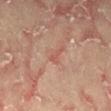workup = no biopsy performed (imaged during a skin exam)
body site = the lower back
patient = male, aged approximately 65
imaging modality = ~15 mm crop, total-body skin-cancer survey